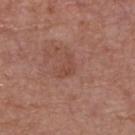Clinical impression:
No biopsy was performed on this lesion — it was imaged during a full skin examination and was not determined to be concerning.
Image and clinical context:
The tile uses white-light illumination. The lesion is located on the front of the torso. The patient is a male aged approximately 65. Automated image analysis of the tile measured a footprint of about 3 mm². It also reported an average lesion color of about L≈46 a*≈23 b*≈28 (CIELAB) and roughly 6 lightness units darker than nearby skin. And it measured a border-irregularity index near 7.5/10 and internal color variation of about 0 on a 0–10 scale. The lesion's longest dimension is about 3 mm. A roughly 15 mm field-of-view crop from a total-body skin photograph.